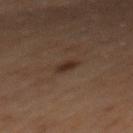No biopsy was performed on this lesion — it was imaged during a full skin examination and was not determined to be concerning. A male patient roughly 70 years of age. Captured under cross-polarized illumination. From the mid back. The lesion's longest dimension is about 2.5 mm. The lesion-visualizer software estimated an outline eccentricity of about 0.8 (0 = round, 1 = elongated) and a symmetry-axis asymmetry near 0.2. The analysis additionally found a within-lesion color-variation index near 1/10 and peripheral color asymmetry of about 0.5. Cropped from a total-body skin-imaging series; the visible field is about 15 mm.